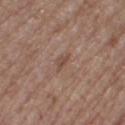{"biopsy_status": "not biopsied; imaged during a skin examination", "automated_metrics": {"border_irregularity_0_10": 3.5, "color_variation_0_10": 1.5, "peripheral_color_asymmetry": 0.5}, "site": "leg", "image": {"source": "total-body photography crop", "field_of_view_mm": 15}, "patient": {"sex": "female", "age_approx": 65}, "lighting": "white-light", "lesion_size": {"long_diameter_mm_approx": 2.5}}